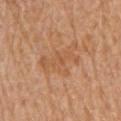  biopsy_status: not biopsied; imaged during a skin examination
  patient:
    sex: female
    age_approx: 55
  site: left upper arm
  image:
    source: total-body photography crop
    field_of_view_mm: 15